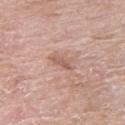imaging modality = 15 mm crop, total-body photography
subject = female, aged around 60
site = the right thigh